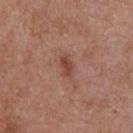follow-up: catalogued during a skin exam; not biopsied
automated lesion analysis: a footprint of about 3 mm², an outline eccentricity of about 0.85 (0 = round, 1 = elongated), and a symmetry-axis asymmetry near 0.3; a lesion–skin lightness drop of about 10 and a normalized border contrast of about 7.5; a color-variation rating of about 1.5/10 and peripheral color asymmetry of about 0.5; a nevus-likeness score of about 30/100 and a detector confidence of about 100 out of 100 that the crop contains a lesion
illumination: white-light illumination
diameter: about 2.5 mm
acquisition: 15 mm crop, total-body photography
anatomic site: the upper back
patient: female, approximately 60 years of age